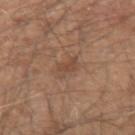workup=total-body-photography surveillance lesion; no biopsy | subject=male, in their 50s | imaging modality=15 mm crop, total-body photography | location=the left forearm | lesion size=about 3 mm | illumination=white-light illumination.